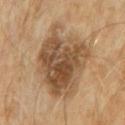The lesion was photographed on a routine skin check and not biopsied; there is no pathology result. A male patient, about 60 years old. A 15 mm close-up tile from a total-body photography series done for melanoma screening. Imaged with cross-polarized lighting. The lesion is on the front of the torso.